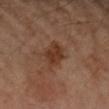{"biopsy_status": "not biopsied; imaged during a skin examination", "site": "arm", "lesion_size": {"long_diameter_mm_approx": 4.0}, "lighting": "cross-polarized", "patient": {"sex": "female", "age_approx": 60}, "image": {"source": "total-body photography crop", "field_of_view_mm": 15}}